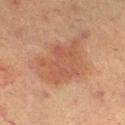{
  "biopsy_status": "not biopsied; imaged during a skin examination",
  "image": {
    "source": "total-body photography crop",
    "field_of_view_mm": 15
  },
  "lesion_size": {
    "long_diameter_mm_approx": 6.0
  },
  "lighting": "cross-polarized",
  "site": "leg",
  "automated_metrics": {
    "cielab_L": 43,
    "cielab_a": 19,
    "cielab_b": 27,
    "vs_skin_contrast_norm": 5.0,
    "color_variation_0_10": 3.0,
    "peripheral_color_asymmetry": 1.0,
    "nevus_likeness_0_100": 0,
    "lesion_detection_confidence_0_100": 100
  },
  "patient": {
    "sex": "female",
    "age_approx": 55
  }
}